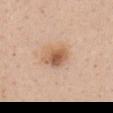No biopsy was performed on this lesion — it was imaged during a full skin examination and was not determined to be concerning. Located on the mid back. The subject is a female aged approximately 50. Automated tile analysis of the lesion measured an area of roughly 8.5 mm², an eccentricity of roughly 0.7, and a shape-asymmetry score of about 0.15 (0 = symmetric). And it measured a nevus-likeness score of about 90/100 and lesion-presence confidence of about 100/100. Cropped from a total-body skin-imaging series; the visible field is about 15 mm. This is a white-light tile. Measured at roughly 4 mm in maximum diameter.